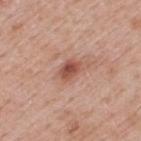Assessment:
Recorded during total-body skin imaging; not selected for excision or biopsy.
Acquisition and patient details:
Imaged with white-light lighting. On the upper back. The lesion-visualizer software estimated a footprint of about 5 mm² and two-axis asymmetry of about 0.2. The analysis additionally found a normalized border contrast of about 8. And it measured an automated nevus-likeness rating near 80 out of 100. A male subject, aged around 40. A 15 mm close-up tile from a total-body photography series done for melanoma screening.